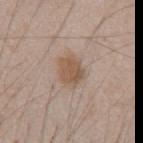Imaged during a routine full-body skin examination; the lesion was not biopsied and no histopathology is available.
A male patient, approximately 45 years of age.
A 15 mm crop from a total-body photograph taken for skin-cancer surveillance.
Automated image analysis of the tile measured border irregularity of about 2 on a 0–10 scale, a within-lesion color-variation index near 2.5/10, and a peripheral color-asymmetry measure near 1. And it measured an automated nevus-likeness rating near 90 out of 100.
The lesion is located on the abdomen.
Measured at roughly 3.5 mm in maximum diameter.
Imaged with white-light lighting.